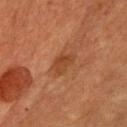Impression:
Imaged during a routine full-body skin examination; the lesion was not biopsied and no histopathology is available.
Image and clinical context:
The patient is a male aged 73–77. A 15 mm close-up tile from a total-body photography series done for melanoma screening. Captured under cross-polarized illumination. Located on the chest. About 3.5 mm across.